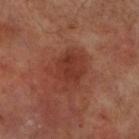{
  "biopsy_status": "not biopsied; imaged during a skin examination",
  "image": {
    "source": "total-body photography crop",
    "field_of_view_mm": 15
  },
  "site": "right lower leg",
  "patient": {
    "sex": "male",
    "age_approx": 70
  },
  "lesion_size": {
    "long_diameter_mm_approx": 4.5
  },
  "lighting": "cross-polarized",
  "automated_metrics": {
    "area_mm2_approx": 13.0,
    "eccentricity": 0.6,
    "shape_asymmetry": 0.35,
    "color_variation_0_10": 2.5,
    "peripheral_color_asymmetry": 1.0
  }
}